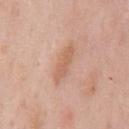Part of a total-body skin-imaging series; this lesion was reviewed on a skin check and was not flagged for biopsy.
The patient is a male aged 53–57.
On the chest.
A roughly 15 mm field-of-view crop from a total-body skin photograph.
Approximately 5 mm at its widest.
Imaged with white-light lighting.